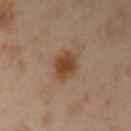  biopsy_status: not biopsied; imaged during a skin examination
  patient:
    sex: female
    age_approx: 40
  site: arm
  automated_metrics:
    cielab_L: 45
    cielab_a: 20
    cielab_b: 32
    vs_skin_darker_L: 10.0
    vs_skin_contrast_norm: 9.0
    nevus_likeness_0_100: 85
    lesion_detection_confidence_0_100: 100
  lighting: cross-polarized
  image:
    source: total-body photography crop
    field_of_view_mm: 15